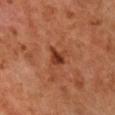biopsy_status: not biopsied; imaged during a skin examination
lesion_size:
  long_diameter_mm_approx: 2.5
site: chest
image:
  source: total-body photography crop
  field_of_view_mm: 15
lighting: cross-polarized
patient:
  sex: female
  age_approx: 60